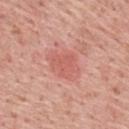lesion size: about 4.5 mm
anatomic site: the upper back
imaging modality: ~15 mm tile from a whole-body skin photo
automated metrics: a footprint of about 11 mm², a shape eccentricity near 0.65, and two-axis asymmetry of about 0.2; a lesion color around L≈60 a*≈29 b*≈28 in CIELAB, a lesion–skin lightness drop of about 7, and a lesion-to-skin contrast of about 5 (normalized; higher = more distinct)
patient: male, approximately 60 years of age